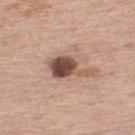follow-up — imaged on a skin check; not biopsied | image — total-body-photography crop, ~15 mm field of view | automated lesion analysis — a lesion area of about 9.5 mm², a shape eccentricity near 0.8, and a symmetry-axis asymmetry near 0.4; a border-irregularity rating of about 4.5/10 and a color-variation rating of about 6.5/10; a nevus-likeness score of about 35/100 and a lesion-detection confidence of about 100/100 | illumination — white-light | patient — male, about 75 years old | location — the upper back.